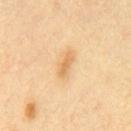notes: catalogued during a skin exam; not biopsied | illumination: cross-polarized | image: ~15 mm tile from a whole-body skin photo | lesion diameter: ~3 mm (longest diameter) | anatomic site: the chest | automated lesion analysis: an area of roughly 4 mm², an outline eccentricity of about 0.9 (0 = round, 1 = elongated), and a symmetry-axis asymmetry near 0.3; about 9 CIELAB-L* units darker than the surrounding skin; border irregularity of about 3 on a 0–10 scale, internal color variation of about 2 on a 0–10 scale, and a peripheral color-asymmetry measure near 0.5 | subject: male, roughly 40 years of age.